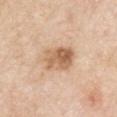Captured during whole-body skin photography for melanoma surveillance; the lesion was not biopsied. A 15 mm crop from a total-body photograph taken for skin-cancer surveillance. A male patient, aged 78 to 82. The lesion is located on the right upper arm. Captured under white-light illumination. The lesion's longest dimension is about 4.5 mm.